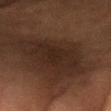Assessment:
The lesion was tiled from a total-body skin photograph and was not biopsied.
Background:
Captured under cross-polarized illumination. From the right forearm. This image is a 15 mm lesion crop taken from a total-body photograph. A female subject, aged approximately 80. The lesion's longest dimension is about 5.5 mm.